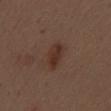Captured during whole-body skin photography for melanoma surveillance; the lesion was not biopsied.
Approximately 4 mm at its widest.
A male subject, aged around 70.
A lesion tile, about 15 mm wide, cut from a 3D total-body photograph.
Captured under white-light illumination.
From the mid back.